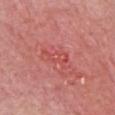notes: catalogued during a skin exam; not biopsied | image source: ~15 mm crop, total-body skin-cancer survey | location: the chest | subject: male, about 80 years old.